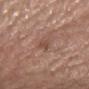Recorded during total-body skin imaging; not selected for excision or biopsy. The lesion is located on the right thigh. The tile uses white-light illumination. A 15 mm crop from a total-body photograph taken for skin-cancer surveillance. Measured at roughly 2.5 mm in maximum diameter. A male subject, about 70 years old. The total-body-photography lesion software estimated a lesion area of about 3 mm², an eccentricity of roughly 0.85, and a symmetry-axis asymmetry near 0.25. The software also gave border irregularity of about 2.5 on a 0–10 scale, internal color variation of about 1 on a 0–10 scale, and a peripheral color-asymmetry measure near 0.